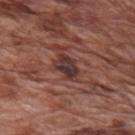Captured during whole-body skin photography for melanoma surveillance; the lesion was not biopsied. The lesion is located on the arm. A male subject aged approximately 75. An algorithmic analysis of the crop reported an area of roughly 6 mm² and a symmetry-axis asymmetry near 0.25. The software also gave a mean CIELAB color near L≈34 a*≈20 b*≈21 and about 11 CIELAB-L* units darker than the surrounding skin. It also reported a border-irregularity rating of about 3/10 and a within-lesion color-variation index near 6.5/10. And it measured a classifier nevus-likeness of about 0/100 and lesion-presence confidence of about 100/100. A 15 mm close-up extracted from a 3D total-body photography capture.